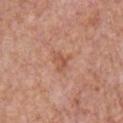The lesion was photographed on a routine skin check and not biopsied; there is no pathology result. The lesion-visualizer software estimated a border-irregularity rating of about 4.5/10, a within-lesion color-variation index near 3/10, and radial color variation of about 1. The software also gave a nevus-likeness score of about 0/100 and a detector confidence of about 100 out of 100 that the crop contains a lesion. A male patient roughly 65 years of age. The lesion is on the front of the torso. Cropped from a total-body skin-imaging series; the visible field is about 15 mm. About 2.5 mm across.